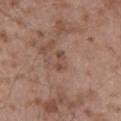No biopsy was performed on this lesion — it was imaged during a full skin examination and was not determined to be concerning. Captured under white-light illumination. On the back. The patient is a male in their mid- to late 50s. A close-up tile cropped from a whole-body skin photograph, about 15 mm across. Automated tile analysis of the lesion measured an area of roughly 2.5 mm² and two-axis asymmetry of about 0.45. It also reported a mean CIELAB color near L≈47 a*≈19 b*≈27 and a normalized lesion–skin contrast near 6.5. The software also gave a nevus-likeness score of about 0/100 and a lesion-detection confidence of about 100/100. About 2.5 mm across.